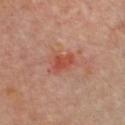Assessment: No biopsy was performed on this lesion — it was imaged during a full skin examination and was not determined to be concerning. Image and clinical context: The subject is a female roughly 60 years of age. A region of skin cropped from a whole-body photographic capture, roughly 15 mm wide. The lesion is located on the chest.